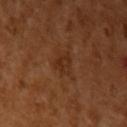workup = no biopsy performed (imaged during a skin exam)
automated lesion analysis = a lesion area of about 4.5 mm² and a shape eccentricity near 0.6; a mean CIELAB color near L≈30 a*≈22 b*≈32 and roughly 6 lightness units darker than nearby skin; border irregularity of about 3.5 on a 0–10 scale
patient = female, roughly 55 years of age
tile lighting = cross-polarized
lesion size = ≈3 mm
anatomic site = the left upper arm
image = ~15 mm tile from a whole-body skin photo